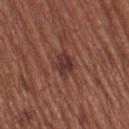Findings:
– biopsy status: imaged on a skin check; not biopsied
– image: ~15 mm tile from a whole-body skin photo
– lesion diameter: about 3.5 mm
– subject: female, aged approximately 55
– automated lesion analysis: an area of roughly 6 mm², a shape eccentricity near 0.65, and a shape-asymmetry score of about 0.15 (0 = symmetric); a mean CIELAB color near L≈35 a*≈22 b*≈23, roughly 9 lightness units darker than nearby skin, and a normalized lesion–skin contrast near 8.5
– illumination: white-light
– location: the left thigh